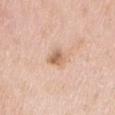{
  "biopsy_status": "not biopsied; imaged during a skin examination",
  "lighting": "white-light",
  "automated_metrics": {
    "cielab_L": 64,
    "cielab_a": 20,
    "cielab_b": 32,
    "vs_skin_darker_L": 11.0,
    "vs_skin_contrast_norm": 7.5,
    "border_irregularity_0_10": 2.5,
    "color_variation_0_10": 4.0,
    "peripheral_color_asymmetry": 1.5
  },
  "patient": {
    "sex": "male",
    "age_approx": 65
  },
  "image": {
    "source": "total-body photography crop",
    "field_of_view_mm": 15
  },
  "lesion_size": {
    "long_diameter_mm_approx": 2.5
  },
  "site": "mid back"
}